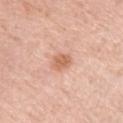Q: Was this lesion biopsied?
A: imaged on a skin check; not biopsied
Q: What is the imaging modality?
A: total-body-photography crop, ~15 mm field of view
Q: Patient demographics?
A: female, aged around 65
Q: Illumination type?
A: white-light illumination
Q: Automated lesion metrics?
A: an area of roughly 4.5 mm² and a shape-asymmetry score of about 0.2 (0 = symmetric); a border-irregularity rating of about 1.5/10, internal color variation of about 1.5 on a 0–10 scale, and peripheral color asymmetry of about 0.5
Q: What is the anatomic site?
A: the left upper arm
Q: What is the lesion's diameter?
A: ≈2.5 mm